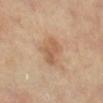{
  "biopsy_status": "not biopsied; imaged during a skin examination",
  "image": {
    "source": "total-body photography crop",
    "field_of_view_mm": 15
  },
  "lesion_size": {
    "long_diameter_mm_approx": 4.0
  },
  "site": "left lower leg",
  "patient": {
    "sex": "female",
    "age_approx": 65
  }
}